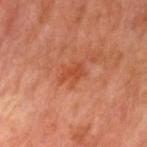Assessment: Imaged during a routine full-body skin examination; the lesion was not biopsied and no histopathology is available. Context: From the left upper arm. A male subject aged 63–67. This image is a 15 mm lesion crop taken from a total-body photograph.